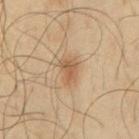Assessment: Imaged during a routine full-body skin examination; the lesion was not biopsied and no histopathology is available. Background: A region of skin cropped from a whole-body photographic capture, roughly 15 mm wide. On the mid back. The lesion's longest dimension is about 3.5 mm. This is a cross-polarized tile. An algorithmic analysis of the crop reported two-axis asymmetry of about 0.25. It also reported a lesion–skin lightness drop of about 9 and a normalized border contrast of about 7. The software also gave border irregularity of about 2.5 on a 0–10 scale, a color-variation rating of about 3/10, and radial color variation of about 1. It also reported a nevus-likeness score of about 85/100. A male subject roughly 65 years of age.